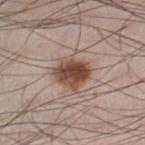Q: Is there a histopathology result?
A: catalogued during a skin exam; not biopsied
Q: Where on the body is the lesion?
A: the left thigh
Q: Patient demographics?
A: male, aged around 35
Q: How was the tile lit?
A: white-light
Q: What is the lesion's diameter?
A: ~4 mm (longest diameter)
Q: How was this image acquired?
A: ~15 mm crop, total-body skin-cancer survey